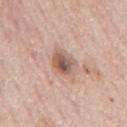From the mid back. A lesion tile, about 15 mm wide, cut from a 3D total-body photograph. A male subject, in their mid-70s. Captured under white-light illumination. The lesion-visualizer software estimated a footprint of about 6.5 mm², an outline eccentricity of about 0.45 (0 = round, 1 = elongated), and a shape-asymmetry score of about 0.2 (0 = symmetric). The analysis additionally found roughly 13 lightness units darker than nearby skin and a normalized lesion–skin contrast near 8.5. Approximately 3 mm at its widest.